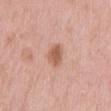Clinical impression:
Part of a total-body skin-imaging series; this lesion was reviewed on a skin check and was not flagged for biopsy.
Context:
This image is a 15 mm lesion crop taken from a total-body photograph. Located on the mid back. A female subject, approximately 60 years of age. This is a white-light tile.